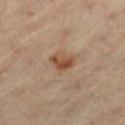Recorded during total-body skin imaging; not selected for excision or biopsy.
A close-up tile cropped from a whole-body skin photograph, about 15 mm across.
A male subject in their 60s.
The lesion is on the left thigh.
Approximately 3 mm at its widest.
Imaged with cross-polarized lighting.